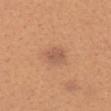{
  "biopsy_status": "not biopsied; imaged during a skin examination",
  "lighting": "white-light",
  "automated_metrics": {
    "area_mm2_approx": 4.0,
    "eccentricity": 0.6,
    "shape_asymmetry": 0.25,
    "cielab_L": 56,
    "cielab_a": 22,
    "cielab_b": 32,
    "vs_skin_darker_L": 8.0,
    "vs_skin_contrast_norm": 6.0,
    "border_irregularity_0_10": 2.5,
    "peripheral_color_asymmetry": 0.5
  },
  "site": "head or neck",
  "image": {
    "source": "total-body photography crop",
    "field_of_view_mm": 15
  },
  "patient": {
    "sex": "female",
    "age_approx": 25
  }
}